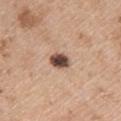  biopsy_status: not biopsied; imaged during a skin examination
  site: upper back
  patient:
    sex: female
    age_approx: 45
  image:
    source: total-body photography crop
    field_of_view_mm: 15
  lesion_size:
    long_diameter_mm_approx: 2.5
  lighting: white-light